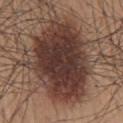Q: What kind of image is this?
A: total-body-photography crop, ~15 mm field of view
Q: What did automated image analysis measure?
A: a border-irregularity index near 2.5/10 and radial color variation of about 1.5
Q: What is the anatomic site?
A: the mid back
Q: Patient demographics?
A: male, aged 63 to 67
Q: What lighting was used for the tile?
A: white-light
Q: Lesion size?
A: ~10 mm (longest diameter)